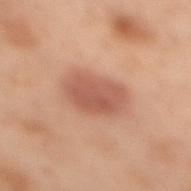Imaged during a routine full-body skin examination; the lesion was not biopsied and no histopathology is available. Approximately 5.5 mm at its widest. A 15 mm crop from a total-body photograph taken for skin-cancer surveillance. The lesion is located on the upper back. Imaged with cross-polarized lighting. The subject is a female in their mid- to late 50s.